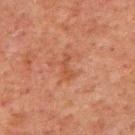{"biopsy_status": "not biopsied; imaged during a skin examination", "patient": {"sex": "male", "age_approx": 60}, "site": "upper back", "image": {"source": "total-body photography crop", "field_of_view_mm": 15}, "automated_metrics": {"eccentricity": 0.95, "shape_asymmetry": 0.4, "cielab_L": 38, "cielab_a": 22, "cielab_b": 29, "border_irregularity_0_10": 5.0, "color_variation_0_10": 0.0, "peripheral_color_asymmetry": 0.0, "nevus_likeness_0_100": 0, "lesion_detection_confidence_0_100": 100}, "lighting": "cross-polarized", "lesion_size": {"long_diameter_mm_approx": 3.0}}